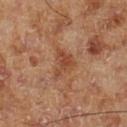{
  "biopsy_status": "not biopsied; imaged during a skin examination",
  "lighting": "cross-polarized",
  "lesion_size": {
    "long_diameter_mm_approx": 4.0
  },
  "image": {
    "source": "total-body photography crop",
    "field_of_view_mm": 15
  },
  "patient": {
    "sex": "male",
    "age_approx": 70
  },
  "site": "left lower leg"
}